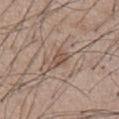<case>
  <biopsy_status>not biopsied; imaged during a skin examination</biopsy_status>
  <patient>
    <sex>male</sex>
    <age_approx>55</age_approx>
  </patient>
  <lesion_size>
    <long_diameter_mm_approx>3.0</long_diameter_mm_approx>
  </lesion_size>
  <site>front of the torso</site>
  <image>
    <source>total-body photography crop</source>
    <field_of_view_mm>15</field_of_view_mm>
  </image>
</case>